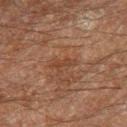{
  "biopsy_status": "not biopsied; imaged during a skin examination",
  "patient": {
    "sex": "male",
    "age_approx": 60
  },
  "site": "right thigh",
  "image": {
    "source": "total-body photography crop",
    "field_of_view_mm": 15
  }
}